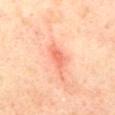image=total-body-photography crop, ~15 mm field of view | patient=female, aged around 60 | lighting=cross-polarized illumination | location=the mid back.